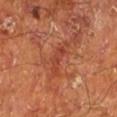No biopsy was performed on this lesion — it was imaged during a full skin examination and was not determined to be concerning. This is a cross-polarized tile. A male patient, approximately 65 years of age. A roughly 15 mm field-of-view crop from a total-body skin photograph. On the left lower leg. The recorded lesion diameter is about 4.5 mm.